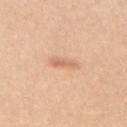<record>
  <biopsy_status>not biopsied; imaged during a skin examination</biopsy_status>
  <lesion_size>
    <long_diameter_mm_approx>2.5</long_diameter_mm_approx>
  </lesion_size>
  <automated_metrics>
    <vs_skin_darker_L>9.0</vs_skin_darker_L>
    <vs_skin_contrast_norm>5.5</vs_skin_contrast_norm>
    <nevus_likeness_0_100>80</nevus_likeness_0_100>
    <lesion_detection_confidence_0_100>100</lesion_detection_confidence_0_100>
  </automated_metrics>
  <patient>
    <sex>female</sex>
    <age_approx>25</age_approx>
  </patient>
  <lighting>white-light</lighting>
  <site>mid back</site>
  <image>
    <source>total-body photography crop</source>
    <field_of_view_mm>15</field_of_view_mm>
  </image>
</record>